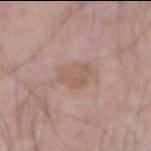This lesion was catalogued during total-body skin photography and was not selected for biopsy. Measured at roughly 3.5 mm in maximum diameter. This image is a 15 mm lesion crop taken from a total-body photograph. Located on the abdomen. A male patient roughly 70 years of age. This is a white-light tile.